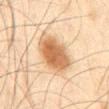Context:
A male subject roughly 45 years of age. The recorded lesion diameter is about 5.5 mm. Located on the abdomen. The lesion-visualizer software estimated a shape eccentricity near 0.65 and a symmetry-axis asymmetry near 0.1. The tile uses cross-polarized illumination. A 15 mm crop from a total-body photograph taken for skin-cancer surveillance.